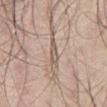Clinical summary: A roughly 15 mm field-of-view crop from a total-body skin photograph. Measured at roughly 2.5 mm in maximum diameter. From the abdomen. A male subject in their mid- to late 50s. The lesion-visualizer software estimated a mean CIELAB color near L≈58 a*≈13 b*≈24, roughly 9 lightness units darker than nearby skin, and a lesion-to-skin contrast of about 6 (normalized; higher = more distinct).